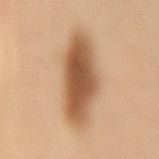Assessment: The lesion was photographed on a routine skin check and not biopsied; there is no pathology result. Clinical summary: A female subject in their mid- to late 60s. The tile uses cross-polarized illumination. The lesion is located on the lower back. A close-up tile cropped from a whole-body skin photograph, about 15 mm across.